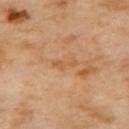Q: Was this lesion biopsied?
A: no biopsy performed (imaged during a skin exam)
Q: Automated lesion metrics?
A: an eccentricity of roughly 0.95 and two-axis asymmetry of about 0.45; a lesion-to-skin contrast of about 5.5 (normalized; higher = more distinct); a border-irregularity index near 5.5/10 and a color-variation rating of about 0/10; a classifier nevus-likeness of about 0/100 and a detector confidence of about 95 out of 100 that the crop contains a lesion
Q: Illumination type?
A: cross-polarized illumination
Q: What is the anatomic site?
A: the upper back
Q: How was this image acquired?
A: 15 mm crop, total-body photography